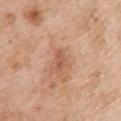This lesion was catalogued during total-body skin photography and was not selected for biopsy.